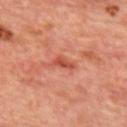- notes — total-body-photography surveillance lesion; no biopsy
- lesion size — ~2.5 mm (longest diameter)
- lighting — cross-polarized illumination
- TBP lesion metrics — an area of roughly 2.5 mm², an outline eccentricity of about 0.9 (0 = round, 1 = elongated), and two-axis asymmetry of about 0.3; an average lesion color of about L≈50 a*≈33 b*≈34 (CIELAB), about 10 CIELAB-L* units darker than the surrounding skin, and a lesion-to-skin contrast of about 7.5 (normalized; higher = more distinct)
- body site — the upper back
- patient — female, in their mid- to late 40s
- acquisition — ~15 mm tile from a whole-body skin photo